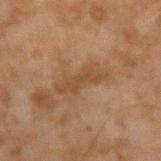follow-up=total-body-photography surveillance lesion; no biopsy | size=≈5.5 mm | subject=male, in their mid-40s | lighting=cross-polarized | location=the arm | acquisition=~15 mm tile from a whole-body skin photo.